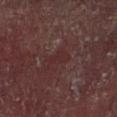Imaged during a routine full-body skin examination; the lesion was not biopsied and no histopathology is available.
The total-body-photography lesion software estimated a mean CIELAB color near L≈23 a*≈18 b*≈16, about 4 CIELAB-L* units darker than the surrounding skin, and a lesion-to-skin contrast of about 5 (normalized; higher = more distinct). The analysis additionally found a within-lesion color-variation index near 0.5/10 and peripheral color asymmetry of about 0.
Cropped from a total-body skin-imaging series; the visible field is about 15 mm.
The subject is a male roughly 55 years of age.
On the right upper arm.